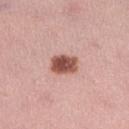| key | value |
|---|---|
| biopsy status | catalogued during a skin exam; not biopsied |
| diameter | about 3 mm |
| tile lighting | white-light |
| acquisition | total-body-photography crop, ~15 mm field of view |
| location | the right lower leg |
| automated lesion analysis | an area of roughly 7 mm² and a shape eccentricity near 0.65; a lesion color around L≈52 a*≈25 b*≈26 in CIELAB; a border-irregularity index near 2/10 and a color-variation rating of about 4.5/10 |
| patient | female, aged approximately 35 |